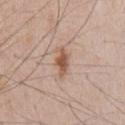• notes · no biopsy performed (imaged during a skin exam)
• automated metrics · a border-irregularity rating of about 3.5/10, internal color variation of about 3 on a 0–10 scale, and a peripheral color-asymmetry measure near 0.5; a nevus-likeness score of about 95/100
• patient · male, aged approximately 65
• lighting · white-light
• location · the front of the torso
• image · total-body-photography crop, ~15 mm field of view